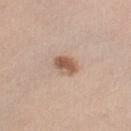biopsy status = imaged on a skin check; not biopsied.